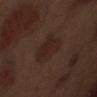Findings:
* workup: total-body-photography surveillance lesion; no biopsy
* illumination: white-light
* automated lesion analysis: an eccentricity of roughly 0.7 and a shape-asymmetry score of about 0.25 (0 = symmetric); a lesion color around L≈23 a*≈16 b*≈20 in CIELAB, roughly 5 lightness units darker than nearby skin, and a normalized border contrast of about 6.5
* lesion size: ≈4.5 mm
* image source: ~15 mm tile from a whole-body skin photo
* location: the abdomen
* patient: male, aged 68 to 72